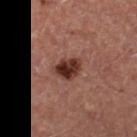Impression: Captured during whole-body skin photography for melanoma surveillance; the lesion was not biopsied. Clinical summary: A female patient, aged 43–47. The lesion is on the left thigh. Automated tile analysis of the lesion measured a mean CIELAB color near L≈33 a*≈22 b*≈24, a lesion–skin lightness drop of about 14, and a lesion-to-skin contrast of about 12 (normalized; higher = more distinct). The software also gave a border-irregularity rating of about 2/10, a within-lesion color-variation index near 5.5/10, and radial color variation of about 2. The analysis additionally found a nevus-likeness score of about 95/100 and a detector confidence of about 100 out of 100 that the crop contains a lesion. A lesion tile, about 15 mm wide, cut from a 3D total-body photograph. The lesion's longest dimension is about 3.5 mm. Captured under cross-polarized illumination.